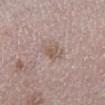workup = catalogued during a skin exam; not biopsied
image = total-body-photography crop, ~15 mm field of view
patient = female, about 65 years old
site = the right lower leg